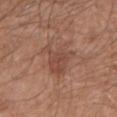notes = total-body-photography surveillance lesion; no biopsy | site = the front of the torso | illumination = white-light | acquisition = 15 mm crop, total-body photography | lesion size = ~5 mm (longest diameter) | image-analysis metrics = an automated nevus-likeness rating near 5 out of 100 and a lesion-detection confidence of about 100/100 | subject = male, in their mid-60s.